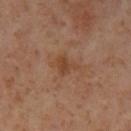– body site: the left lower leg
– size: ~2.5 mm (longest diameter)
– image source: ~15 mm tile from a whole-body skin photo
– illumination: cross-polarized illumination
– automated lesion analysis: a mean CIELAB color near L≈44 a*≈21 b*≈32 and a lesion-to-skin contrast of about 6 (normalized; higher = more distinct); a border-irregularity rating of about 3.5/10 and a within-lesion color-variation index near 1.5/10; a detector confidence of about 100 out of 100 that the crop contains a lesion
– patient: female, approximately 55 years of age